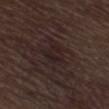Q: Patient demographics?
A: male, roughly 70 years of age
Q: What is the imaging modality?
A: ~15 mm tile from a whole-body skin photo
Q: What is the anatomic site?
A: the left lower leg
Q: Illumination type?
A: white-light illumination